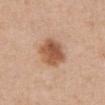– location: the abdomen
– image: 15 mm crop, total-body photography
– diameter: about 4 mm
– subject: female, approximately 40 years of age
– automated metrics: a border-irregularity rating of about 1.5/10, internal color variation of about 4.5 on a 0–10 scale, and peripheral color asymmetry of about 1.5; lesion-presence confidence of about 100/100
– tile lighting: white-light